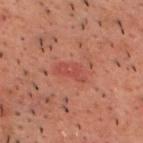Captured during whole-body skin photography for melanoma surveillance; the lesion was not biopsied.
Longest diameter approximately 3.5 mm.
Captured under cross-polarized illumination.
The lesion is located on the head or neck.
A male subject, aged approximately 50.
A close-up tile cropped from a whole-body skin photograph, about 15 mm across.